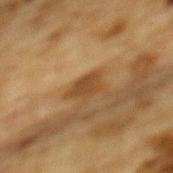Q: Is there a histopathology result?
A: total-body-photography surveillance lesion; no biopsy
Q: What is the imaging modality?
A: total-body-photography crop, ~15 mm field of view
Q: Patient demographics?
A: male, in their mid-80s or older
Q: What lighting was used for the tile?
A: cross-polarized illumination
Q: What is the anatomic site?
A: the mid back
Q: How large is the lesion?
A: ~3.5 mm (longest diameter)